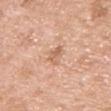Part of a total-body skin-imaging series; this lesion was reviewed on a skin check and was not flagged for biopsy.
The total-body-photography lesion software estimated peripheral color asymmetry of about 0.5.
Measured at roughly 2.5 mm in maximum diameter.
From the chest.
A close-up tile cropped from a whole-body skin photograph, about 15 mm across.
The subject is a male roughly 65 years of age.
Captured under white-light illumination.